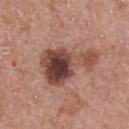Impression: Part of a total-body skin-imaging series; this lesion was reviewed on a skin check and was not flagged for biopsy. Acquisition and patient details: The tile uses white-light illumination. A lesion tile, about 15 mm wide, cut from a 3D total-body photograph. The lesion's longest dimension is about 7 mm. Located on the chest. A male subject aged around 70.